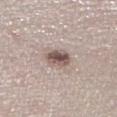Clinical summary: A region of skin cropped from a whole-body photographic capture, roughly 15 mm wide. The lesion is on the right lower leg. A male subject about 60 years old.